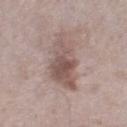workup: catalogued during a skin exam; not biopsied | body site: the right lower leg | lesion diameter: ~8 mm (longest diameter) | patient: male, about 75 years old | illumination: white-light | image-analysis metrics: an eccentricity of roughly 0.9; a nevus-likeness score of about 45/100 and lesion-presence confidence of about 95/100 | imaging modality: total-body-photography crop, ~15 mm field of view.